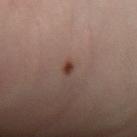Recorded during total-body skin imaging; not selected for excision or biopsy. The lesion-visualizer software estimated a lesion area of about 2 mm². The analysis additionally found an automated nevus-likeness rating near 95 out of 100. Longest diameter approximately 2 mm. On the left forearm. This is a cross-polarized tile. This image is a 15 mm lesion crop taken from a total-body photograph. A male subject aged around 55.